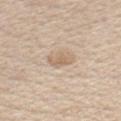  biopsy_status: not biopsied; imaged during a skin examination
  patient:
    sex: male
    age_approx: 70
  lesion_size:
    long_diameter_mm_approx: 3.0
  image:
    source: total-body photography crop
    field_of_view_mm: 15
  site: chest
  automated_metrics:
    vs_skin_darker_L: 9.0
    peripheral_color_asymmetry: 0.5
    nevus_likeness_0_100: 50
    lesion_detection_confidence_0_100: 100